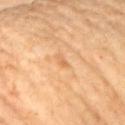notes — no biopsy performed (imaged during a skin exam) | anatomic site — the arm | lesion diameter — ≈1.5 mm | subject — female, in their mid-60s | image source — 15 mm crop, total-body photography.